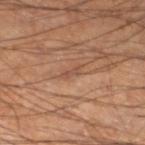This is a cross-polarized tile. Cropped from a whole-body photographic skin survey; the tile spans about 15 mm. From the left lower leg. Automated tile analysis of the lesion measured a border-irregularity index near 4/10. A male patient aged around 60.